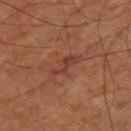Impression: No biopsy was performed on this lesion — it was imaged during a full skin examination and was not determined to be concerning. Image and clinical context: Cropped from a whole-body photographic skin survey; the tile spans about 15 mm. The subject is aged approximately 65. Automated tile analysis of the lesion measured roughly 7 lightness units darker than nearby skin and a normalized lesion–skin contrast near 6.5. The analysis additionally found border irregularity of about 6 on a 0–10 scale and radial color variation of about 0.5. Approximately 4 mm at its widest. The lesion is located on the right thigh. This is a cross-polarized tile.